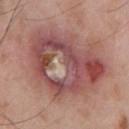Clinical impression:
No biopsy was performed on this lesion — it was imaged during a full skin examination and was not determined to be concerning.
Context:
Imaged with white-light lighting. The patient is a male aged 63 to 67. Automated image analysis of the tile measured a border-irregularity rating of about 3.5/10, a color-variation rating of about 10/10, and a peripheral color-asymmetry measure near 4.5. The analysis additionally found lesion-presence confidence of about 90/100. This image is a 15 mm lesion crop taken from a total-body photograph. Located on the chest.